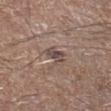Case summary:
- follow-up — total-body-photography surveillance lesion; no biopsy
- size — ~3 mm (longest diameter)
- image source — 15 mm crop, total-body photography
- patient — male, aged 63 to 67
- lighting — white-light illumination
- location — the right lower leg